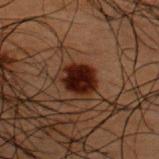Recorded during total-body skin imaging; not selected for excision or biopsy. Cropped from a whole-body photographic skin survey; the tile spans about 15 mm. Located on the upper back. Automated tile analysis of the lesion measured an area of roughly 9 mm², a shape eccentricity near 0.45, and two-axis asymmetry of about 0.15. And it measured an average lesion color of about L≈14 a*≈18 b*≈17 (CIELAB), a lesion–skin lightness drop of about 13, and a normalized lesion–skin contrast near 16. The software also gave a border-irregularity index near 1/10 and internal color variation of about 4 on a 0–10 scale. It also reported a detector confidence of about 100 out of 100 that the crop contains a lesion. Captured under cross-polarized illumination. A male subject aged 48–52.